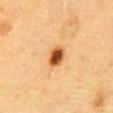{"biopsy_status": "not biopsied; imaged during a skin examination", "patient": {"sex": "male", "age_approx": "85+"}, "image": {"source": "total-body photography crop", "field_of_view_mm": 15}, "lesion_size": {"long_diameter_mm_approx": 2.5}, "site": "abdomen", "lighting": "cross-polarized"}